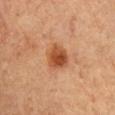This lesion was catalogued during total-body skin photography and was not selected for biopsy.
The lesion's longest dimension is about 3.5 mm.
The subject is a female aged 43–47.
Located on the arm.
The total-body-photography lesion software estimated an outline eccentricity of about 0.4 (0 = round, 1 = elongated) and two-axis asymmetry of about 0.2.
A 15 mm close-up extracted from a 3D total-body photography capture.
Imaged with cross-polarized lighting.